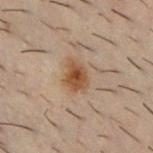{"biopsy_status": "not biopsied; imaged during a skin examination", "lighting": "cross-polarized", "site": "chest", "image": {"source": "total-body photography crop", "field_of_view_mm": 15}, "patient": {"sex": "male", "age_approx": 30}, "lesion_size": {"long_diameter_mm_approx": 3.5}}